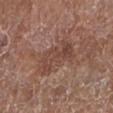workup: no biopsy performed (imaged during a skin exam)
illumination: white-light illumination
image-analysis metrics: a footprint of about 11 mm², an outline eccentricity of about 0.85 (0 = round, 1 = elongated), and a symmetry-axis asymmetry near 0.35; a border-irregularity index near 5.5/10, a within-lesion color-variation index near 3.5/10, and radial color variation of about 1.5
subject: female, roughly 80 years of age
acquisition: total-body-photography crop, ~15 mm field of view
body site: the left lower leg
diameter: ≈5.5 mm